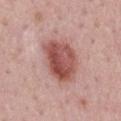Located on the mid back.
Automated tile analysis of the lesion measured a lesion area of about 18 mm², a shape eccentricity near 0.5, and a shape-asymmetry score of about 0.2 (0 = symmetric). The analysis additionally found a border-irregularity rating of about 2/10, a within-lesion color-variation index near 7/10, and a peripheral color-asymmetry measure near 2.5.
A 15 mm close-up extracted from a 3D total-body photography capture.
The tile uses white-light illumination.
A male subject aged 48–52.